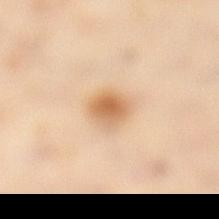| feature | finding |
|---|---|
| subject | female, approximately 45 years of age |
| image source | ~15 mm crop, total-body skin-cancer survey |
| anatomic site | the left lower leg |